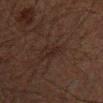  biopsy_status: not biopsied; imaged during a skin examination
  patient:
    sex: male
    age_approx: 60
  automated_metrics:
    area_mm2_approx: 3.0
    shape_asymmetry: 0.45
    border_irregularity_0_10: 4.5
    peripheral_color_asymmetry: 0.5
  lighting: cross-polarized
  site: mid back
  image:
    source: total-body photography crop
    field_of_view_mm: 15